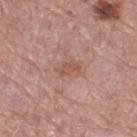Part of a total-body skin-imaging series; this lesion was reviewed on a skin check and was not flagged for biopsy.
The patient is a female approximately 55 years of age.
This is a white-light tile.
A 15 mm crop from a total-body photograph taken for skin-cancer surveillance.
On the right thigh.
The recorded lesion diameter is about 3 mm.